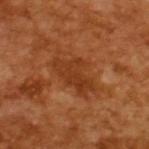{"biopsy_status": "not biopsied; imaged during a skin examination", "image": {"source": "total-body photography crop", "field_of_view_mm": 15}, "patient": {"sex": "male", "age_approx": 65}}